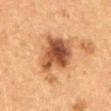Assessment:
Recorded during total-body skin imaging; not selected for excision or biopsy.
Acquisition and patient details:
The recorded lesion diameter is about 7 mm. The patient is a female about 50 years old. Cropped from a total-body skin-imaging series; the visible field is about 15 mm. The total-body-photography lesion software estimated a footprint of about 21 mm², an outline eccentricity of about 0.75 (0 = round, 1 = elongated), and a symmetry-axis asymmetry near 0.4. The analysis additionally found roughly 13 lightness units darker than nearby skin and a lesion-to-skin contrast of about 10 (normalized; higher = more distinct). And it measured a nevus-likeness score of about 55/100 and a detector confidence of about 100 out of 100 that the crop contains a lesion. Captured under cross-polarized illumination. The lesion is located on the abdomen.